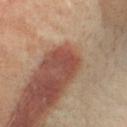| feature | finding |
|---|---|
| image | ~15 mm tile from a whole-body skin photo |
| diameter | about 3.5 mm |
| lighting | cross-polarized illumination |
| TBP lesion metrics | an outline eccentricity of about 0.75 (0 = round, 1 = elongated); lesion-presence confidence of about 95/100 |
| subject | female, approximately 75 years of age |
| location | the head or neck |